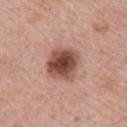{
  "biopsy_status": "not biopsied; imaged during a skin examination",
  "automated_metrics": {
    "eccentricity": 0.45,
    "shape_asymmetry": 0.15,
    "cielab_L": 49,
    "cielab_a": 23,
    "cielab_b": 26,
    "vs_skin_contrast_norm": 11.5,
    "border_irregularity_0_10": 1.5,
    "peripheral_color_asymmetry": 1.5
  },
  "patient": {
    "sex": "male",
    "age_approx": 65
  },
  "lighting": "white-light",
  "site": "right upper arm",
  "image": {
    "source": "total-body photography crop",
    "field_of_view_mm": 15
  }
}